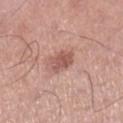Q: Was a biopsy performed?
A: catalogued during a skin exam; not biopsied
Q: Who is the patient?
A: male, roughly 40 years of age
Q: What kind of image is this?
A: total-body-photography crop, ~15 mm field of view
Q: What is the anatomic site?
A: the right lower leg